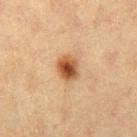Q: Is there a histopathology result?
A: total-body-photography surveillance lesion; no biopsy
Q: What is the lesion's diameter?
A: about 3 mm
Q: Lesion location?
A: the leg
Q: How was the tile lit?
A: cross-polarized
Q: What did automated image analysis measure?
A: a lesion area of about 6.5 mm² and a symmetry-axis asymmetry near 0.15; a lesion color around L≈46 a*≈20 b*≈34 in CIELAB, roughly 14 lightness units darker than nearby skin, and a lesion-to-skin contrast of about 10.5 (normalized; higher = more distinct); an automated nevus-likeness rating near 100 out of 100
Q: What kind of image is this?
A: 15 mm crop, total-body photography
Q: Patient demographics?
A: female, aged around 40